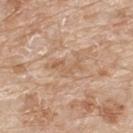Part of a total-body skin-imaging series; this lesion was reviewed on a skin check and was not flagged for biopsy. A 15 mm crop from a total-body photograph taken for skin-cancer surveillance. A male subject aged around 80. The lesion is on the upper back. This is a white-light tile. The recorded lesion diameter is about 4.5 mm.